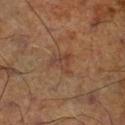notes: total-body-photography surveillance lesion; no biopsy | location: the right lower leg | acquisition: total-body-photography crop, ~15 mm field of view | patient: male, aged approximately 70.